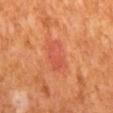Assessment:
The lesion was tiled from a total-body skin photograph and was not biopsied.
Image and clinical context:
On the mid back. Imaged with cross-polarized lighting. Cropped from a whole-body photographic skin survey; the tile spans about 15 mm. The subject is a male in their mid- to late 60s.